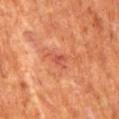Q: How large is the lesion?
A: about 2.5 mm
Q: Illumination type?
A: cross-polarized illumination
Q: How was this image acquired?
A: total-body-photography crop, ~15 mm field of view
Q: Where on the body is the lesion?
A: the mid back
Q: Who is the patient?
A: male, in their mid-60s